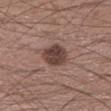notes = total-body-photography surveillance lesion; no biopsy | imaging modality = ~15 mm tile from a whole-body skin photo | subject = male, about 30 years old | body site = the left lower leg.